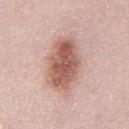biopsy status: no biopsy performed (imaged during a skin exam); subject: male, aged 48 to 52; location: the front of the torso; image: 15 mm crop, total-body photography; illumination: white-light.